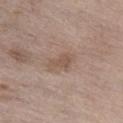Clinical impression:
Imaged during a routine full-body skin examination; the lesion was not biopsied and no histopathology is available.
Image and clinical context:
A close-up tile cropped from a whole-body skin photograph, about 15 mm across. The lesion's longest dimension is about 3 mm. The subject is a male approximately 70 years of age. The lesion is located on the leg.